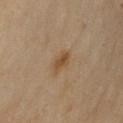biopsy_status: not biopsied; imaged during a skin examination